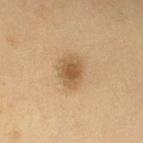<record>
  <biopsy_status>not biopsied; imaged during a skin examination</biopsy_status>
  <site>left upper arm</site>
  <lesion_size>
    <long_diameter_mm_approx>3.5</long_diameter_mm_approx>
  </lesion_size>
  <lighting>cross-polarized</lighting>
  <image>
    <source>total-body photography crop</source>
    <field_of_view_mm>15</field_of_view_mm>
  </image>
  <patient>
    <sex>male</sex>
    <age_approx>60</age_approx>
  </patient>
</record>